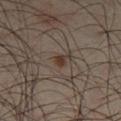• biopsy status: total-body-photography surveillance lesion; no biopsy
• automated metrics: a border-irregularity rating of about 1/10, a within-lesion color-variation index near 0/10, and a peripheral color-asymmetry measure near 0; a classifier nevus-likeness of about 90/100 and a detector confidence of about 100 out of 100 that the crop contains a lesion
• body site: the left lower leg
• lesion size: ≈1.5 mm
• imaging modality: ~15 mm crop, total-body skin-cancer survey
• patient: male, aged around 45
• illumination: cross-polarized illumination